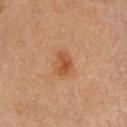Background: Approximately 3 mm at its widest. A lesion tile, about 15 mm wide, cut from a 3D total-body photograph. Imaged with cross-polarized lighting. From the head or neck. A female patient roughly 45 years of age. An algorithmic analysis of the crop reported an automated nevus-likeness rating near 80 out of 100 and lesion-presence confidence of about 100/100.